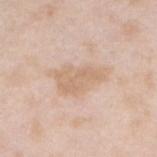The lesion was tiled from a total-body skin photograph and was not biopsied. Measured at roughly 6 mm in maximum diameter. A close-up tile cropped from a whole-body skin photograph, about 15 mm across. The total-body-photography lesion software estimated a mean CIELAB color near L≈68 a*≈16 b*≈31, roughly 7 lightness units darker than nearby skin, and a lesion-to-skin contrast of about 5.5 (normalized; higher = more distinct). And it measured border irregularity of about 4.5 on a 0–10 scale, a color-variation rating of about 2/10, and a peripheral color-asymmetry measure near 0.5. And it measured a nevus-likeness score of about 0/100 and a lesion-detection confidence of about 100/100. A male subject, aged 43 to 47. Captured under white-light illumination. The lesion is on the right forearm.